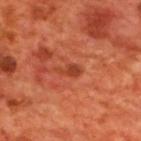Clinical impression:
Imaged during a routine full-body skin examination; the lesion was not biopsied and no histopathology is available.
Acquisition and patient details:
The recorded lesion diameter is about 3 mm. The lesion is located on the upper back. The patient is a male aged around 70. A lesion tile, about 15 mm wide, cut from a 3D total-body photograph. The tile uses cross-polarized illumination. Automated tile analysis of the lesion measured a footprint of about 3 mm², an outline eccentricity of about 0.9 (0 = round, 1 = elongated), and a symmetry-axis asymmetry near 0.45. It also reported border irregularity of about 4.5 on a 0–10 scale, a within-lesion color-variation index near 1.5/10, and a peripheral color-asymmetry measure near 0.5. It also reported a classifier nevus-likeness of about 0/100 and a lesion-detection confidence of about 100/100.